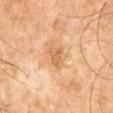biopsy status — no biopsy performed (imaged during a skin exam)
patient — male, roughly 60 years of age
location — the right leg
image — 15 mm crop, total-body photography
automated lesion analysis — a footprint of about 4 mm² and an outline eccentricity of about 0.75 (0 = round, 1 = elongated); a lesion color around L≈61 a*≈22 b*≈39 in CIELAB, roughly 9 lightness units darker than nearby skin, and a normalized lesion–skin contrast near 6; border irregularity of about 3.5 on a 0–10 scale and internal color variation of about 2 on a 0–10 scale; a lesion-detection confidence of about 100/100